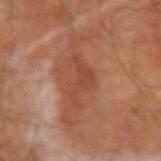This lesion was catalogued during total-body skin photography and was not selected for biopsy. A male subject aged approximately 65. A 15 mm crop from a total-body photograph taken for skin-cancer surveillance. About 5 mm across. Located on the right forearm. Imaged with cross-polarized lighting. The lesion-visualizer software estimated an eccentricity of roughly 0.85 and two-axis asymmetry of about 0.65. The analysis additionally found a border-irregularity rating of about 8/10, a color-variation rating of about 2/10, and a peripheral color-asymmetry measure near 0.5. The software also gave a lesion-detection confidence of about 100/100.